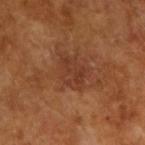The lesion was tiled from a total-body skin photograph and was not biopsied. Cropped from a total-body skin-imaging series; the visible field is about 15 mm. Approximately 4 mm at its widest. A male subject, approximately 65 years of age. The total-body-photography lesion software estimated a footprint of about 8 mm², a shape eccentricity near 0.7, and a symmetry-axis asymmetry near 0.3. The analysis additionally found a lesion color around L≈36 a*≈23 b*≈30 in CIELAB and a lesion–skin lightness drop of about 6. The analysis additionally found a border-irregularity rating of about 3.5/10, a within-lesion color-variation index near 2.5/10, and peripheral color asymmetry of about 1. It also reported an automated nevus-likeness rating near 0 out of 100.